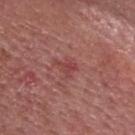Clinical impression: Imaged during a routine full-body skin examination; the lesion was not biopsied and no histopathology is available. Acquisition and patient details: Located on the head or neck. A male patient aged 73 to 77. Longest diameter approximately 2.5 mm. The tile uses white-light illumination. Cropped from a whole-body photographic skin survey; the tile spans about 15 mm.